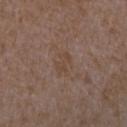{
  "biopsy_status": "not biopsied; imaged during a skin examination",
  "lighting": "white-light",
  "site": "right forearm",
  "patient": {
    "sex": "female",
    "age_approx": 35
  },
  "image": {
    "source": "total-body photography crop",
    "field_of_view_mm": 15
  },
  "automated_metrics": {
    "eccentricity": 0.8,
    "shape_asymmetry": 0.4,
    "border_irregularity_0_10": 5.5,
    "color_variation_0_10": 1.0
  }
}